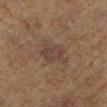<case>
  <biopsy_status>not biopsied; imaged during a skin examination</biopsy_status>
  <lighting>cross-polarized</lighting>
  <site>left lower leg</site>
  <lesion_size>
    <long_diameter_mm_approx>4.0</long_diameter_mm_approx>
  </lesion_size>
  <image>
    <source>total-body photography crop</source>
    <field_of_view_mm>15</field_of_view_mm>
  </image>
  <patient>
    <sex>male</sex>
    <age_approx>85+</age_approx>
  </patient>
</case>